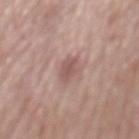Impression:
This lesion was catalogued during total-body skin photography and was not selected for biopsy.
Background:
Cropped from a whole-body photographic skin survey; the tile spans about 15 mm. On the mid back. A male subject, aged 63 to 67. Imaged with white-light lighting.